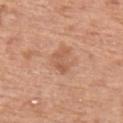{
  "biopsy_status": "not biopsied; imaged during a skin examination",
  "site": "right upper arm",
  "image": {
    "source": "total-body photography crop",
    "field_of_view_mm": 15
  },
  "patient": {
    "sex": "male",
    "age_approx": 65
  }
}